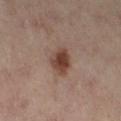{
  "biopsy_status": "not biopsied; imaged during a skin examination",
  "lighting": "cross-polarized",
  "automated_metrics": {
    "area_mm2_approx": 7.0,
    "eccentricity": 0.45,
    "shape_asymmetry": 0.2,
    "nevus_likeness_0_100": 100,
    "lesion_detection_confidence_0_100": 100
  },
  "image": {
    "source": "total-body photography crop",
    "field_of_view_mm": 15
  },
  "lesion_size": {
    "long_diameter_mm_approx": 3.0
  },
  "site": "left leg",
  "patient": {
    "sex": "female",
    "age_approx": 55
  }
}